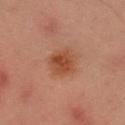Assessment:
No biopsy was performed on this lesion — it was imaged during a full skin examination and was not determined to be concerning.
Image and clinical context:
On the head or neck. A female patient about 20 years old. The tile uses cross-polarized illumination. A close-up tile cropped from a whole-body skin photograph, about 15 mm across. Longest diameter approximately 3 mm.